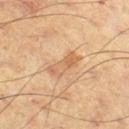follow-up: catalogued during a skin exam; not biopsied
body site: the left thigh
lesion diameter: ≈4 mm
lighting: cross-polarized
subject: male, in their mid-60s
image: ~15 mm crop, total-body skin-cancer survey